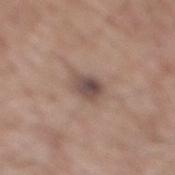Assessment:
The lesion was photographed on a routine skin check and not biopsied; there is no pathology result.
Acquisition and patient details:
A male subject in their 60s. A lesion tile, about 15 mm wide, cut from a 3D total-body photograph. The lesion is located on the mid back.